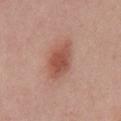biopsy status: catalogued during a skin exam; not biopsied
patient: male, approximately 60 years of age
TBP lesion metrics: a footprint of about 12 mm², a shape eccentricity near 0.8, and a symmetry-axis asymmetry near 0.15; a mean CIELAB color near L≈53 a*≈25 b*≈28, about 11 CIELAB-L* units darker than the surrounding skin, and a normalized lesion–skin contrast near 8; internal color variation of about 3.5 on a 0–10 scale and a peripheral color-asymmetry measure near 1; an automated nevus-likeness rating near 100 out of 100 and a lesion-detection confidence of about 100/100
body site: the chest
size: ~5 mm (longest diameter)
image: total-body-photography crop, ~15 mm field of view